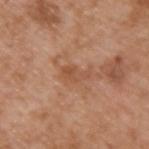Captured during whole-body skin photography for melanoma surveillance; the lesion was not biopsied. The tile uses white-light illumination. A 15 mm close-up extracted from a 3D total-body photography capture. A male subject, aged 63 to 67. The lesion is located on the upper back.